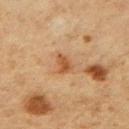Assessment: Imaged during a routine full-body skin examination; the lesion was not biopsied and no histopathology is available. Image and clinical context: A female patient approximately 45 years of age. On the arm. A close-up tile cropped from a whole-body skin photograph, about 15 mm across. Approximately 2.5 mm at its widest. Captured under cross-polarized illumination.